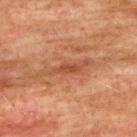No biopsy was performed on this lesion — it was imaged during a full skin examination and was not determined to be concerning. The lesion is on the back. A 15 mm close-up tile from a total-body photography series done for melanoma screening. Captured under cross-polarized illumination. The recorded lesion diameter is about 3 mm. The subject is a male roughly 75 years of age.